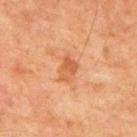This lesion was catalogued during total-body skin photography and was not selected for biopsy. Located on the mid back. Measured at roughly 3 mm in maximum diameter. Automated image analysis of the tile measured roughly 7 lightness units darker than nearby skin and a normalized lesion–skin contrast near 6.5. And it measured a classifier nevus-likeness of about 20/100 and lesion-presence confidence of about 100/100. A 15 mm crop from a total-body photograph taken for skin-cancer surveillance. A male subject, in their 80s. Imaged with cross-polarized lighting.